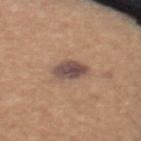{"biopsy_status": "not biopsied; imaged during a skin examination", "site": "upper back", "image": {"source": "total-body photography crop", "field_of_view_mm": 15}, "lesion_size": {"long_diameter_mm_approx": 3.5}, "patient": {"sex": "female", "age_approx": 35}}